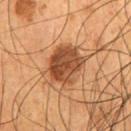Impression: The lesion was tiled from a total-body skin photograph and was not biopsied. Image and clinical context: The lesion is located on the front of the torso. Automated tile analysis of the lesion measured an average lesion color of about L≈45 a*≈23 b*≈34 (CIELAB), roughly 13 lightness units darker than nearby skin, and a normalized lesion–skin contrast near 10. A lesion tile, about 15 mm wide, cut from a 3D total-body photograph. The subject is a male in their mid- to late 50s.